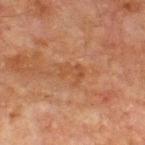The lesion was tiled from a total-body skin photograph and was not biopsied.
This image is a 15 mm lesion crop taken from a total-body photograph.
A male patient approximately 65 years of age.
About 2.5 mm across.
Captured under cross-polarized illumination.
The lesion is located on the chest.